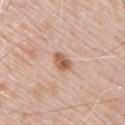follow-up = imaged on a skin check; not biopsied
image source = ~15 mm crop, total-body skin-cancer survey
illumination = white-light illumination
TBP lesion metrics = a color-variation rating of about 4.5/10 and a peripheral color-asymmetry measure near 1.5
patient = male, aged approximately 70
site = the upper back
diameter = ~3 mm (longest diameter)